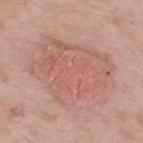follow-up — catalogued during a skin exam; not biopsied
imaging modality — ~15 mm tile from a whole-body skin photo
anatomic site — the upper back
patient — male, aged 53 to 57